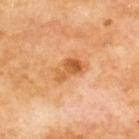Impression: The lesion was photographed on a routine skin check and not biopsied; there is no pathology result. Background: A male subject roughly 70 years of age. This is a cross-polarized tile. Located on the upper back. A 15 mm close-up tile from a total-body photography series done for melanoma screening.